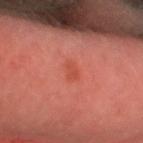  lighting: cross-polarized
  site: head or neck
  image:
    source: total-body photography crop
    field_of_view_mm: 15
  patient:
    sex: male
    age_approx: 30
  lesion_size:
    long_diameter_mm_approx: 2.5